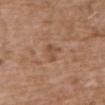notes: total-body-photography surveillance lesion; no biopsy | lighting: white-light | imaging modality: ~15 mm crop, total-body skin-cancer survey | subject: female, roughly 75 years of age | body site: the mid back | automated metrics: a lesion color around L≈50 a*≈21 b*≈31 in CIELAB, roughly 7 lightness units darker than nearby skin, and a lesion-to-skin contrast of about 5.5 (normalized; higher = more distinct) | lesion diameter: about 3 mm.